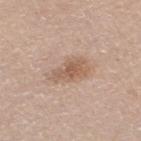Recorded during total-body skin imaging; not selected for excision or biopsy. A female subject, aged approximately 45. A close-up tile cropped from a whole-body skin photograph, about 15 mm across. From the left thigh.